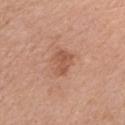{
  "biopsy_status": "not biopsied; imaged during a skin examination",
  "image": {
    "source": "total-body photography crop",
    "field_of_view_mm": 15
  },
  "lesion_size": {
    "long_diameter_mm_approx": 3.0
  },
  "site": "arm",
  "lighting": "white-light",
  "patient": {
    "sex": "female",
    "age_approx": 60
  }
}